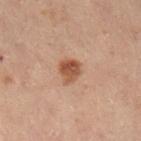• image source · total-body-photography crop, ~15 mm field of view
• subject · male, aged approximately 55
• diameter · ~2.5 mm (longest diameter)
• anatomic site · the right lower leg
• automated metrics · a border-irregularity rating of about 2/10 and a within-lesion color-variation index near 4.5/10
• tile lighting · cross-polarized illumination